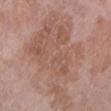{
  "lighting": "white-light",
  "image": {
    "source": "total-body photography crop",
    "field_of_view_mm": 15
  },
  "patient": {
    "sex": "female",
    "age_approx": 70
  },
  "lesion_size": {
    "long_diameter_mm_approx": 13.0
  },
  "automated_metrics": {
    "area_mm2_approx": 55.0,
    "eccentricity": 0.85,
    "shape_asymmetry": 0.4,
    "cielab_L": 55,
    "cielab_a": 19,
    "cielab_b": 26,
    "border_irregularity_0_10": 7.0,
    "peripheral_color_asymmetry": 1.0
  },
  "site": "leg"
}